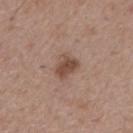biopsy_status: not biopsied; imaged during a skin examination
lesion_size:
  long_diameter_mm_approx: 3.0
image:
  source: total-body photography crop
  field_of_view_mm: 15
automated_metrics:
  area_mm2_approx: 6.5
  eccentricity: 0.65
  shape_asymmetry: 0.3
  cielab_L: 48
  cielab_a: 18
  cielab_b: 26
  vs_skin_darker_L: 10.0
  vs_skin_contrast_norm: 7.5
patient:
  sex: male
  age_approx: 55
lighting: white-light